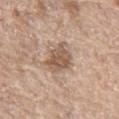The lesion was photographed on a routine skin check and not biopsied; there is no pathology result. This image is a 15 mm lesion crop taken from a total-body photograph. The total-body-photography lesion software estimated a footprint of about 8 mm², a shape eccentricity near 0.55, and two-axis asymmetry of about 0.3. It also reported a lesion–skin lightness drop of about 12 and a normalized border contrast of about 8. It also reported a border-irregularity index near 3/10. The patient is a female aged approximately 60. The tile uses white-light illumination. The lesion is on the left thigh. The recorded lesion diameter is about 3.5 mm.